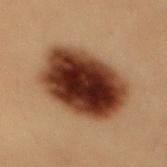The lesion was photographed on a routine skin check and not biopsied; there is no pathology result. A 15 mm close-up tile from a total-body photography series done for melanoma screening. The lesion is on the mid back. Captured under cross-polarized illumination. A female patient, aged around 20. Approximately 8 mm at its widest.